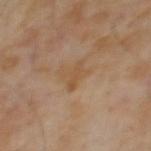biopsy status: total-body-photography surveillance lesion; no biopsy | imaging modality: total-body-photography crop, ~15 mm field of view | patient: male, approximately 65 years of age | lesion diameter: ~3 mm (longest diameter) | image-analysis metrics: a mean CIELAB color near L≈49 a*≈17 b*≈33 and a lesion-to-skin contrast of about 5.5 (normalized; higher = more distinct); a border-irregularity rating of about 3.5/10 and peripheral color asymmetry of about 0.5 | tile lighting: cross-polarized illumination.